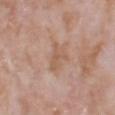- workup — catalogued during a skin exam; not biopsied
- patient — male, aged 68–72
- illumination — white-light
- TBP lesion metrics — a mean CIELAB color near L≈57 a*≈19 b*≈29, roughly 7 lightness units darker than nearby skin, and a lesion-to-skin contrast of about 5.5 (normalized; higher = more distinct); a lesion-detection confidence of about 100/100
- site — the head or neck
- image source — ~15 mm crop, total-body skin-cancer survey